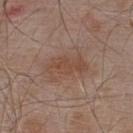Context: A male subject, roughly 55 years of age. A close-up tile cropped from a whole-body skin photograph, about 15 mm across. This is a white-light tile. On the upper back. The lesion's longest dimension is about 5 mm.